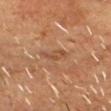Part of a total-body skin-imaging series; this lesion was reviewed on a skin check and was not flagged for biopsy.
A roughly 15 mm field-of-view crop from a total-body skin photograph.
Measured at roughly 3 mm in maximum diameter.
A male subject, in their mid-50s.
On the head or neck.
Automated tile analysis of the lesion measured an area of roughly 3.5 mm², a shape eccentricity near 0.85, and a shape-asymmetry score of about 0.45 (0 = symmetric). And it measured an average lesion color of about L≈46 a*≈21 b*≈31 (CIELAB), about 7 CIELAB-L* units darker than the surrounding skin, and a normalized lesion–skin contrast near 5.5.